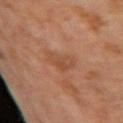Q: Is there a histopathology result?
A: catalogued during a skin exam; not biopsied
Q: Who is the patient?
A: male, about 65 years old
Q: What is the imaging modality?
A: 15 mm crop, total-body photography
Q: What is the anatomic site?
A: the right upper arm